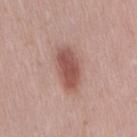notes=catalogued during a skin exam; not biopsied | patient=female, approximately 40 years of age | acquisition=~15 mm crop, total-body skin-cancer survey | anatomic site=the right thigh.